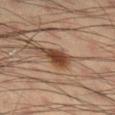Q: Is there a histopathology result?
A: catalogued during a skin exam; not biopsied
Q: How was the tile lit?
A: cross-polarized
Q: Who is the patient?
A: male, approximately 35 years of age
Q: What is the anatomic site?
A: the right lower leg
Q: What is the imaging modality?
A: total-body-photography crop, ~15 mm field of view
Q: What did automated image analysis measure?
A: a lesion area of about 5.5 mm² and a shape eccentricity near 0.8; lesion-presence confidence of about 100/100
Q: What is the lesion's diameter?
A: about 3.5 mm